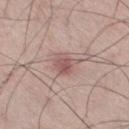Q: Was this lesion biopsied?
A: total-body-photography surveillance lesion; no biopsy
Q: What did automated image analysis measure?
A: an area of roughly 6 mm² and an outline eccentricity of about 0.55 (0 = round, 1 = elongated); an average lesion color of about L≈55 a*≈20 b*≈21 (CIELAB) and a lesion-to-skin contrast of about 6.5 (normalized; higher = more distinct); a border-irregularity index near 3.5/10, a within-lesion color-variation index near 3.5/10, and a peripheral color-asymmetry measure near 1.5
Q: How was this image acquired?
A: total-body-photography crop, ~15 mm field of view
Q: Patient demographics?
A: male, aged approximately 30
Q: What is the anatomic site?
A: the right thigh
Q: How was the tile lit?
A: white-light